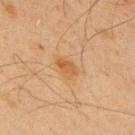Context:
About 3 mm across. The lesion is on the upper back. A male patient aged 63–67. The tile uses cross-polarized illumination. A 15 mm close-up tile from a total-body photography series done for melanoma screening.